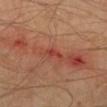Impression: Part of a total-body skin-imaging series; this lesion was reviewed on a skin check and was not flagged for biopsy. Clinical summary: A male subject, in their 40s. Longest diameter approximately 3 mm. Captured under cross-polarized illumination. Cropped from a total-body skin-imaging series; the visible field is about 15 mm. Located on the left lower leg.